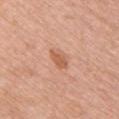Clinical impression:
Recorded during total-body skin imaging; not selected for excision or biopsy.
Image and clinical context:
A female patient, aged around 60. The lesion-visualizer software estimated a mean CIELAB color near L≈60 a*≈24 b*≈34 and a normalized border contrast of about 6.5. And it measured an automated nevus-likeness rating near 40 out of 100 and a lesion-detection confidence of about 100/100. The lesion is located on the chest. This is a white-light tile. About 3 mm across. A region of skin cropped from a whole-body photographic capture, roughly 15 mm wide.